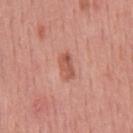Captured during whole-body skin photography for melanoma surveillance; the lesion was not biopsied. A 15 mm close-up extracted from a 3D total-body photography capture. Imaged with white-light lighting. A male patient about 45 years old. The lesion is on the upper back.